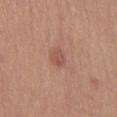This lesion was catalogued during total-body skin photography and was not selected for biopsy.